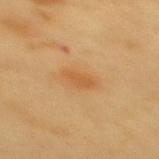Findings:
* patient — male, roughly 60 years of age
* image source — ~15 mm tile from a whole-body skin photo
* tile lighting — cross-polarized
* image-analysis metrics — a footprint of about 4.5 mm², a shape eccentricity near 0.85, and a symmetry-axis asymmetry near 0.25; an automated nevus-likeness rating near 65 out of 100 and lesion-presence confidence of about 100/100
* site — the mid back